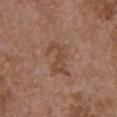Recorded during total-body skin imaging; not selected for excision or biopsy. Automated tile analysis of the lesion measured an average lesion color of about L≈46 a*≈19 b*≈29 (CIELAB), about 7 CIELAB-L* units darker than the surrounding skin, and a normalized lesion–skin contrast near 6. The analysis additionally found border irregularity of about 7 on a 0–10 scale and a color-variation rating of about 1.5/10. It also reported an automated nevus-likeness rating near 0 out of 100 and a lesion-detection confidence of about 100/100. A female subject, roughly 65 years of age. A close-up tile cropped from a whole-body skin photograph, about 15 mm across. Approximately 4.5 mm at its widest. Located on the chest. Imaged with white-light lighting.